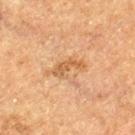biopsy status=catalogued during a skin exam; not biopsied | location=the leg | lesion diameter=~3.5 mm (longest diameter) | acquisition=~15 mm tile from a whole-body skin photo | patient=male, in their mid- to late 70s.